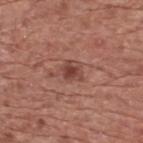workup: total-body-photography surveillance lesion; no biopsy | tile lighting: white-light illumination | subject: male, approximately 75 years of age | location: the upper back | imaging modality: ~15 mm crop, total-body skin-cancer survey.